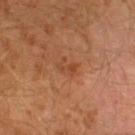The lesion was tiled from a total-body skin photograph and was not biopsied. The lesion is located on the left lower leg. A male subject, aged approximately 30. Automated tile analysis of the lesion measured a footprint of about 4 mm², an outline eccentricity of about 0.7 (0 = round, 1 = elongated), and a shape-asymmetry score of about 0.4 (0 = symmetric). A region of skin cropped from a whole-body photographic capture, roughly 15 mm wide.